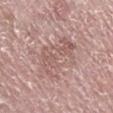Q: Lesion size?
A: ≈5.5 mm
Q: Illumination type?
A: white-light illumination
Q: What is the imaging modality?
A: ~15 mm tile from a whole-body skin photo
Q: Patient demographics?
A: male, approximately 75 years of age
Q: What is the anatomic site?
A: the right lower leg